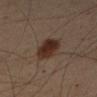Impression:
Recorded during total-body skin imaging; not selected for excision or biopsy.
Acquisition and patient details:
The tile uses cross-polarized illumination. Cropped from a whole-body photographic skin survey; the tile spans about 15 mm. Automated tile analysis of the lesion measured an area of roughly 9.5 mm² and a symmetry-axis asymmetry near 0.15. It also reported a border-irregularity index near 1.5/10 and a color-variation rating of about 3.5/10. From the right upper arm. The patient is a male aged around 50. Measured at roughly 4 mm in maximum diameter.